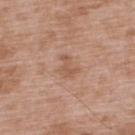| field | value |
|---|---|
| lesion size | ~2.5 mm (longest diameter) |
| subject | male, aged around 50 |
| anatomic site | the upper back |
| acquisition | 15 mm crop, total-body photography |
| automated lesion analysis | a shape eccentricity near 0.7 and two-axis asymmetry of about 0.4; a classifier nevus-likeness of about 0/100 and a detector confidence of about 100 out of 100 that the crop contains a lesion |